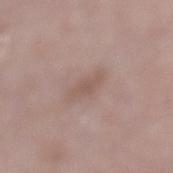subject: female, in their 70s
lesion diameter: about 3.5 mm
location: the left lower leg
imaging modality: total-body-photography crop, ~15 mm field of view
automated lesion analysis: an outline eccentricity of about 0.9 (0 = round, 1 = elongated) and a symmetry-axis asymmetry near 0.2; an automated nevus-likeness rating near 0 out of 100 and lesion-presence confidence of about 100/100